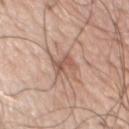{
  "biopsy_status": "not biopsied; imaged during a skin examination",
  "patient": {
    "sex": "male",
    "age_approx": 70
  },
  "automated_metrics": {
    "area_mm2_approx": 4.5,
    "eccentricity": 0.5,
    "shape_asymmetry": 0.35
  },
  "image": {
    "source": "total-body photography crop",
    "field_of_view_mm": 15
  },
  "lesion_size": {
    "long_diameter_mm_approx": 3.0
  },
  "lighting": "white-light",
  "site": "chest"
}